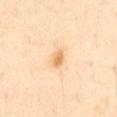Case summary:
• workup · total-body-photography surveillance lesion; no biopsy
• site · the abdomen
• TBP lesion metrics · an area of roughly 3 mm², an outline eccentricity of about 0.7 (0 = round, 1 = elongated), and two-axis asymmetry of about 0.25; a nevus-likeness score of about 90/100
• lighting · cross-polarized
• patient · male, about 55 years old
• image source · ~15 mm tile from a whole-body skin photo
• diameter · about 2 mm